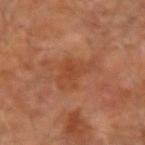Impression: Recorded during total-body skin imaging; not selected for excision or biopsy.